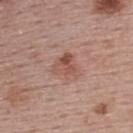Recorded during total-body skin imaging; not selected for excision or biopsy. Automated tile analysis of the lesion measured a lesion color around L≈52 a*≈22 b*≈26 in CIELAB, a lesion–skin lightness drop of about 9, and a lesion-to-skin contrast of about 6.5 (normalized; higher = more distinct). The software also gave a color-variation rating of about 6.5/10 and peripheral color asymmetry of about 2.5. The tile uses white-light illumination. A 15 mm crop from a total-body photograph taken for skin-cancer surveillance. A female subject aged approximately 55. The lesion is located on the upper back. Measured at roughly 3.5 mm in maximum diameter.